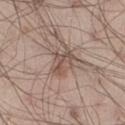– follow-up: catalogued during a skin exam; not biopsied
– lesion size: ≈4 mm
– TBP lesion metrics: a lesion area of about 7.5 mm² and a symmetry-axis asymmetry near 0.3; a lesion color around L≈52 a*≈16 b*≈24 in CIELAB, a lesion–skin lightness drop of about 9, and a lesion-to-skin contrast of about 6.5 (normalized; higher = more distinct); lesion-presence confidence of about 85/100
– patient: male, aged 28 to 32
– lighting: white-light
– acquisition: ~15 mm crop, total-body skin-cancer survey
– location: the leg